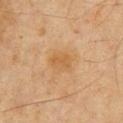Q: Is there a histopathology result?
A: catalogued during a skin exam; not biopsied
Q: What kind of image is this?
A: ~15 mm tile from a whole-body skin photo
Q: What is the anatomic site?
A: the front of the torso
Q: How large is the lesion?
A: ≈3 mm
Q: Patient demographics?
A: male, aged approximately 65
Q: What did automated image analysis measure?
A: about 5 CIELAB-L* units darker than the surrounding skin and a normalized border contrast of about 5.5; border irregularity of about 2 on a 0–10 scale, internal color variation of about 2.5 on a 0–10 scale, and a peripheral color-asymmetry measure near 1
Q: Illumination type?
A: cross-polarized